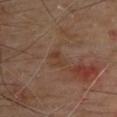Case summary:
• notes · catalogued during a skin exam; not biopsied
• TBP lesion metrics · a border-irregularity index near 4/10 and peripheral color asymmetry of about 1
• patient · male, aged approximately 65
• tile lighting · cross-polarized
• acquisition · total-body-photography crop, ~15 mm field of view
• location · the back
• lesion diameter · about 2.5 mm